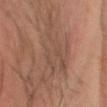Imaged during a routine full-body skin examination; the lesion was not biopsied and no histopathology is available.
The patient is a male in their 60s.
A 15 mm close-up tile from a total-body photography series done for melanoma screening.
The lesion is located on the head or neck.